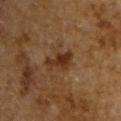Impression:
No biopsy was performed on this lesion — it was imaged during a full skin examination and was not determined to be concerning.
Clinical summary:
A male subject in their mid-60s. On the upper back. Cropped from a whole-body photographic skin survey; the tile spans about 15 mm.